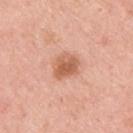notes = catalogued during a skin exam; not biopsied
patient = male, in their mid- to late 60s
diameter = ~3.5 mm (longest diameter)
automated lesion analysis = a mean CIELAB color near L≈60 a*≈25 b*≈34 and a lesion–skin lightness drop of about 12; an automated nevus-likeness rating near 85 out of 100
lighting = white-light
body site = the upper back
imaging modality = total-body-photography crop, ~15 mm field of view